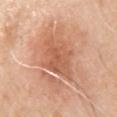patient: male, aged approximately 70 | acquisition: total-body-photography crop, ~15 mm field of view | site: the right upper arm | tile lighting: white-light | size: ~6 mm (longest diameter).